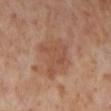follow-up — imaged on a skin check; not biopsied | image-analysis metrics — an eccentricity of roughly 0.5 and two-axis asymmetry of about 0.6; a lesion-detection confidence of about 100/100 | subject — female, aged 53 to 57 | tile lighting — cross-polarized illumination | diameter — ≈4.5 mm | image source — total-body-photography crop, ~15 mm field of view | location — the left lower leg.